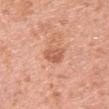Findings:
- biopsy status: total-body-photography surveillance lesion; no biopsy
- subject: female, aged 38 to 42
- acquisition: ~15 mm tile from a whole-body skin photo
- body site: the right upper arm